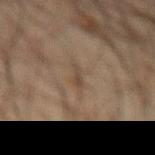This lesion was catalogued during total-body skin photography and was not selected for biopsy.
Cropped from a total-body skin-imaging series; the visible field is about 15 mm.
Automated tile analysis of the lesion measured a lesion area of about 2 mm², an outline eccentricity of about 0.95 (0 = round, 1 = elongated), and two-axis asymmetry of about 0.5. And it measured a lesion color around L≈35 a*≈11 b*≈22 in CIELAB, roughly 6 lightness units darker than nearby skin, and a lesion-to-skin contrast of about 6 (normalized; higher = more distinct). The analysis additionally found a nevus-likeness score of about 0/100 and a lesion-detection confidence of about 70/100.
Measured at roughly 2.5 mm in maximum diameter.
This is a cross-polarized tile.
A male subject, aged 63–67.
The lesion is on the abdomen.